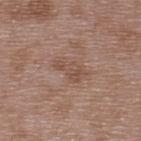biopsy status: imaged on a skin check; not biopsied | patient: male, aged around 50 | illumination: white-light | body site: the back | acquisition: ~15 mm crop, total-body skin-cancer survey | diameter: ~3.5 mm (longest diameter) | image-analysis metrics: an area of roughly 5 mm², an eccentricity of roughly 0.9, and two-axis asymmetry of about 0.45; an automated nevus-likeness rating near 0 out of 100 and lesion-presence confidence of about 100/100.